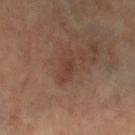<record>
<biopsy_status>not biopsied; imaged during a skin examination</biopsy_status>
<lesion_size>
  <long_diameter_mm_approx>3.5</long_diameter_mm_approx>
</lesion_size>
<automated_metrics>
  <area_mm2_approx>5.0</area_mm2_approx>
  <eccentricity>0.9</eccentricity>
  <shape_asymmetry>0.25</shape_asymmetry>
  <cielab_L>38</cielab_L>
  <cielab_a>19</cielab_a>
  <cielab_b>26</cielab_b>
  <vs_skin_darker_L>6.0</vs_skin_darker_L>
  <vs_skin_contrast_norm>6.0</vs_skin_contrast_norm>
  <peripheral_color_asymmetry>0.5</peripheral_color_asymmetry>
  <nevus_likeness_0_100>5</nevus_likeness_0_100>
  <lesion_detection_confidence_0_100>100</lesion_detection_confidence_0_100>
</automated_metrics>
<site>right leg</site>
<image>
  <source>total-body photography crop</source>
  <field_of_view_mm>15</field_of_view_mm>
</image>
<lighting>cross-polarized</lighting>
<patient>
  <sex>female</sex>
  <age_approx>65</age_approx>
</patient>
</record>